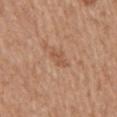| key | value |
|---|---|
| notes | catalogued during a skin exam; not biopsied |
| automated lesion analysis | a border-irregularity index near 3.5/10 and internal color variation of about 1 on a 0–10 scale; a classifier nevus-likeness of about 0/100 and a detector confidence of about 100 out of 100 that the crop contains a lesion |
| acquisition | ~15 mm crop, total-body skin-cancer survey |
| diameter | ≈2.5 mm |
| body site | the mid back |
| subject | male, aged 68–72 |